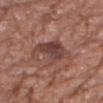Imaged during a routine full-body skin examination; the lesion was not biopsied and no histopathology is available.
The tile uses white-light illumination.
Cropped from a whole-body photographic skin survey; the tile spans about 15 mm.
Automated tile analysis of the lesion measured border irregularity of about 5.5 on a 0–10 scale and peripheral color asymmetry of about 1.5. And it measured a nevus-likeness score of about 0/100 and a detector confidence of about 100 out of 100 that the crop contains a lesion.
The lesion is located on the chest.
A male subject, approximately 70 years of age.